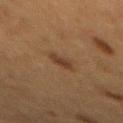<record>
  <biopsy_status>not biopsied; imaged during a skin examination</biopsy_status>
  <patient>
    <sex>female</sex>
    <age_approx>55</age_approx>
  </patient>
  <site>mid back</site>
  <image>
    <source>total-body photography crop</source>
    <field_of_view_mm>15</field_of_view_mm>
  </image>
  <lesion_size>
    <long_diameter_mm_approx>2.5</long_diameter_mm_approx>
  </lesion_size>
  <lighting>cross-polarized</lighting>
</record>